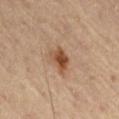<record>
<biopsy_status>not biopsied; imaged during a skin examination</biopsy_status>
<patient>
  <sex>male</sex>
  <age_approx>65</age_approx>
</patient>
<automated_metrics>
  <area_mm2_approx>5.5</area_mm2_approx>
  <shape_asymmetry>0.3</shape_asymmetry>
</automated_metrics>
<site>abdomen</site>
<lesion_size>
  <long_diameter_mm_approx>3.0</long_diameter_mm_approx>
</lesion_size>
<image>
  <source>total-body photography crop</source>
  <field_of_view_mm>15</field_of_view_mm>
</image>
</record>